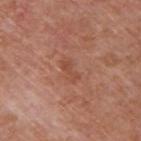Part of a total-body skin-imaging series; this lesion was reviewed on a skin check and was not flagged for biopsy. A roughly 15 mm field-of-view crop from a total-body skin photograph. The lesion is located on the upper back. A male subject aged 63–67. Imaged with white-light lighting. The total-body-photography lesion software estimated a lesion color around L≈48 a*≈25 b*≈31 in CIELAB.